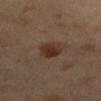<lesion>
  <biopsy_status>not biopsied; imaged during a skin examination</biopsy_status>
  <lesion_size>
    <long_diameter_mm_approx>3.5</long_diameter_mm_approx>
  </lesion_size>
  <lighting>cross-polarized</lighting>
  <image>
    <source>total-body photography crop</source>
    <field_of_view_mm>15</field_of_view_mm>
  </image>
  <patient>
    <sex>female</sex>
    <age_approx>40</age_approx>
  </patient>
  <site>left arm</site>
  <automated_metrics>
    <cielab_L>32</cielab_L>
    <cielab_a>18</cielab_a>
    <cielab_b>24</cielab_b>
    <vs_skin_darker_L>9.0</vs_skin_darker_L>
    <vs_skin_contrast_norm>9.0</vs_skin_contrast_norm>
    <peripheral_color_asymmetry>1.5</peripheral_color_asymmetry>
  </automated_metrics>
</lesion>